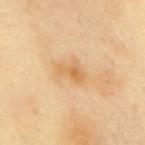biopsy status: no biopsy performed (imaged during a skin exam)
body site: the mid back
illumination: cross-polarized illumination
patient: female, aged approximately 60
lesion diameter: ~3 mm (longest diameter)
acquisition: ~15 mm crop, total-body skin-cancer survey